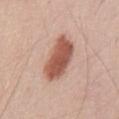Impression: The lesion was tiled from a total-body skin photograph and was not biopsied. Context: On the abdomen. Measured at roughly 6 mm in maximum diameter. Cropped from a total-body skin-imaging series; the visible field is about 15 mm. Imaged with white-light lighting. A male subject in their mid-40s. Automated image analysis of the tile measured about 15 CIELAB-L* units darker than the surrounding skin and a normalized border contrast of about 10.